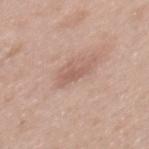| feature | finding |
|---|---|
| follow-up | total-body-photography surveillance lesion; no biopsy |
| image | ~15 mm tile from a whole-body skin photo |
| size | ~2.5 mm (longest diameter) |
| anatomic site | the mid back |
| subject | female, aged 38 to 42 |